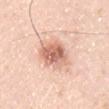Imaged during a routine full-body skin examination; the lesion was not biopsied and no histopathology is available.
About 4.5 mm across.
A roughly 15 mm field-of-view crop from a total-body skin photograph.
From the upper back.
The total-body-photography lesion software estimated a footprint of about 12 mm², an eccentricity of roughly 0.6, and two-axis asymmetry of about 0.15. The analysis additionally found a nevus-likeness score of about 85/100 and a lesion-detection confidence of about 100/100.
A male subject aged approximately 35.